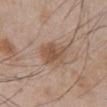A roughly 15 mm field-of-view crop from a total-body skin photograph.
The lesion's longest dimension is about 3.5 mm.
Located on the abdomen.
Imaged with white-light lighting.
A male patient, approximately 45 years of age.